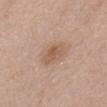biopsy status: imaged on a skin check; not biopsied | site: the abdomen | diameter: ≈3.5 mm | imaging modality: total-body-photography crop, ~15 mm field of view | lighting: white-light | TBP lesion metrics: a lesion area of about 6 mm², an eccentricity of roughly 0.8, and a shape-asymmetry score of about 0.3 (0 = symmetric); a mean CIELAB color near L≈57 a*≈19 b*≈30 and a lesion–skin lightness drop of about 8; an automated nevus-likeness rating near 15 out of 100 and a detector confidence of about 100 out of 100 that the crop contains a lesion | subject: female, aged 63–67.